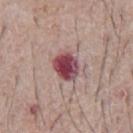Notes:
- notes — total-body-photography surveillance lesion; no biopsy
- acquisition — ~15 mm crop, total-body skin-cancer survey
- anatomic site — the chest
- subject — male, approximately 65 years of age